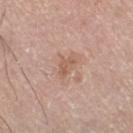workup: no biopsy performed (imaged during a skin exam); patient: male, roughly 75 years of age; body site: the leg; imaging modality: ~15 mm crop, total-body skin-cancer survey.